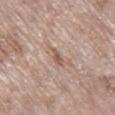The lesion was tiled from a total-body skin photograph and was not biopsied. A female subject roughly 75 years of age. A 15 mm close-up extracted from a 3D total-body photography capture. The lesion is on the right lower leg.